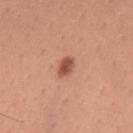A 15 mm close-up extracted from a 3D total-body photography capture. A male patient aged approximately 30. From the mid back. The recorded lesion diameter is about 2.5 mm.